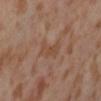{"biopsy_status": "not biopsied; imaged during a skin examination", "site": "abdomen", "lighting": "cross-polarized", "image": {"source": "total-body photography crop", "field_of_view_mm": 15}, "patient": {"sex": "female", "age_approx": 55}}